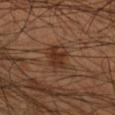subject = male, approximately 55 years of age
tile lighting = cross-polarized illumination
automated metrics = an average lesion color of about L≈30 a*≈18 b*≈26 (CIELAB); a detector confidence of about 100 out of 100 that the crop contains a lesion
anatomic site = the right lower leg
lesion size = about 3.5 mm
acquisition = total-body-photography crop, ~15 mm field of view